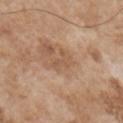Imaged during a routine full-body skin examination; the lesion was not biopsied and no histopathology is available.
Located on the chest.
The patient is a male about 55 years old.
Longest diameter approximately 2.5 mm.
The tile uses white-light illumination.
The lesion-visualizer software estimated a footprint of about 3.5 mm² and a shape eccentricity near 0.75. The software also gave a lesion color around L≈55 a*≈19 b*≈32 in CIELAB, about 7 CIELAB-L* units darker than the surrounding skin, and a normalized lesion–skin contrast near 5. The analysis additionally found an automated nevus-likeness rating near 0 out of 100 and a detector confidence of about 100 out of 100 that the crop contains a lesion.
A 15 mm close-up extracted from a 3D total-body photography capture.